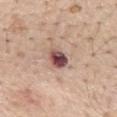Impression:
Imaged during a routine full-body skin examination; the lesion was not biopsied and no histopathology is available.
Clinical summary:
Located on the back. A male subject aged approximately 55. A close-up tile cropped from a whole-body skin photograph, about 15 mm across.